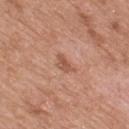Context:
The lesion is on the mid back. Automated image analysis of the tile measured a lesion color around L≈54 a*≈24 b*≈31 in CIELAB and roughly 9 lightness units darker than nearby skin. The software also gave a border-irregularity rating of about 4.5/10, internal color variation of about 1 on a 0–10 scale, and radial color variation of about 0. Measured at roughly 3 mm in maximum diameter. Cropped from a total-body skin-imaging series; the visible field is about 15 mm. The patient is a male aged 48–52.